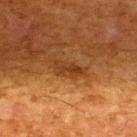Q: Was a biopsy performed?
A: catalogued during a skin exam; not biopsied
Q: Who is the patient?
A: male, approximately 65 years of age
Q: Lesion size?
A: about 3.5 mm
Q: Illumination type?
A: cross-polarized
Q: What is the anatomic site?
A: the upper back
Q: What is the imaging modality?
A: ~15 mm tile from a whole-body skin photo